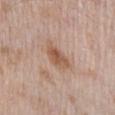Recorded during total-body skin imaging; not selected for excision or biopsy.
On the chest.
A male subject, aged approximately 70.
Cropped from a total-body skin-imaging series; the visible field is about 15 mm.